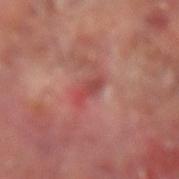This lesion was catalogued during total-body skin photography and was not selected for biopsy. On the right forearm. A male patient approximately 65 years of age. Measured at roughly 3 mm in maximum diameter. A region of skin cropped from a whole-body photographic capture, roughly 15 mm wide.